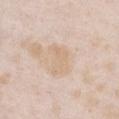Case summary:
• biopsy status — no biopsy performed (imaged during a skin exam)
• acquisition — total-body-photography crop, ~15 mm field of view
• location — the chest
• subject — female, about 25 years old